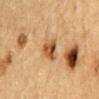* workup: imaged on a skin check; not biopsied
* image-analysis metrics: an area of roughly 6 mm² and an outline eccentricity of about 0.5 (0 = round, 1 = elongated); an average lesion color of about L≈46 a*≈18 b*≈35 (CIELAB), roughly 12 lightness units darker than nearby skin, and a normalized border contrast of about 9; a border-irregularity index near 2.5/10 and a within-lesion color-variation index near 7/10
* anatomic site: the back
* subject: male, aged around 85 or older
* lesion size: about 2.5 mm
* tile lighting: cross-polarized illumination
* imaging modality: ~15 mm tile from a whole-body skin photo